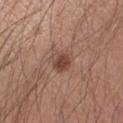Clinical impression:
The lesion was tiled from a total-body skin photograph and was not biopsied.
Context:
Located on the left forearm. The subject is a male about 35 years old. A 15 mm crop from a total-body photograph taken for skin-cancer surveillance.